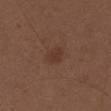The lesion was tiled from a total-body skin photograph and was not biopsied. Captured under white-light illumination. Located on the left upper arm. About 2.5 mm across. An algorithmic analysis of the crop reported a lesion area of about 4.5 mm², an outline eccentricity of about 0.55 (0 = round, 1 = elongated), and a symmetry-axis asymmetry near 0.15. The subject is a male approximately 30 years of age. A 15 mm crop from a total-body photograph taken for skin-cancer surveillance.